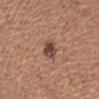{
  "biopsy_status": "not biopsied; imaged during a skin examination",
  "image": {
    "source": "total-body photography crop",
    "field_of_view_mm": 15
  },
  "patient": {
    "sex": "male",
    "age_approx": 50
  },
  "site": "arm"
}